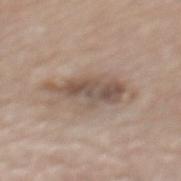Part of a total-body skin-imaging series; this lesion was reviewed on a skin check and was not flagged for biopsy. A roughly 15 mm field-of-view crop from a total-body skin photograph. The subject is a male aged around 75. The tile uses white-light illumination. The lesion is on the back.